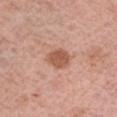Findings:
• biopsy status: total-body-photography surveillance lesion; no biopsy
• lighting: white-light
• subject: female, in their mid-60s
• image-analysis metrics: a lesion area of about 5.5 mm² and two-axis asymmetry of about 0.15; a mean CIELAB color near L≈55 a*≈25 b*≈31, a lesion–skin lightness drop of about 12, and a normalized border contrast of about 8; a border-irregularity index near 1.5/10, a color-variation rating of about 2/10, and peripheral color asymmetry of about 0.5; a lesion-detection confidence of about 100/100
• image: total-body-photography crop, ~15 mm field of view
• diameter: about 2.5 mm
• body site: the arm